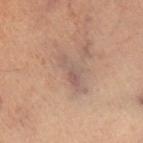{
  "biopsy_status": "not biopsied; imaged during a skin examination"
}